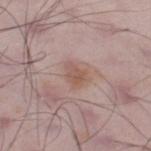Image and clinical context: The patient is a male aged 48–52. Located on the right lower leg. A roughly 15 mm field-of-view crop from a total-body skin photograph. About 3 mm across.